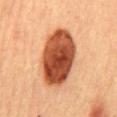Q: Is there a histopathology result?
A: imaged on a skin check; not biopsied
Q: What is the imaging modality?
A: total-body-photography crop, ~15 mm field of view
Q: What is the lesion's diameter?
A: ~8 mm (longest diameter)
Q: What did automated image analysis measure?
A: a border-irregularity index near 1.5/10 and internal color variation of about 7 on a 0–10 scale; a detector confidence of about 100 out of 100 that the crop contains a lesion
Q: What lighting was used for the tile?
A: cross-polarized
Q: What is the anatomic site?
A: the mid back
Q: Patient demographics?
A: female, aged around 60